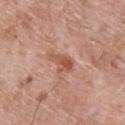Imaged during a routine full-body skin examination; the lesion was not biopsied and no histopathology is available.
The patient is a male in their mid- to late 70s.
This is a white-light tile.
The lesion-visualizer software estimated a lesion area of about 4.5 mm², an outline eccentricity of about 0.85 (0 = round, 1 = elongated), and two-axis asymmetry of about 0.35. It also reported a nevus-likeness score of about 0/100 and a lesion-detection confidence of about 100/100.
The lesion is located on the upper back.
Cropped from a total-body skin-imaging series; the visible field is about 15 mm.